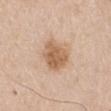Assessment:
No biopsy was performed on this lesion — it was imaged during a full skin examination and was not determined to be concerning.
Clinical summary:
The tile uses white-light illumination. The subject is a male in their mid- to late 60s. A 15 mm close-up extracted from a 3D total-body photography capture. The lesion is located on the left upper arm.